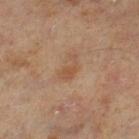{
  "biopsy_status": "not biopsied; imaged during a skin examination",
  "site": "left lower leg",
  "image": {
    "source": "total-body photography crop",
    "field_of_view_mm": 15
  },
  "patient": {
    "sex": "male",
    "age_approx": 60
  }
}